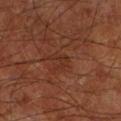Assessment:
Imaged during a routine full-body skin examination; the lesion was not biopsied and no histopathology is available.
Background:
The lesion is on the left lower leg. A male patient, roughly 65 years of age. A region of skin cropped from a whole-body photographic capture, roughly 15 mm wide. The lesion's longest dimension is about 2.5 mm.